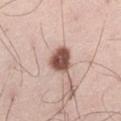Clinical impression:
Imaged during a routine full-body skin examination; the lesion was not biopsied and no histopathology is available.
Context:
Automated tile analysis of the lesion measured a lesion area of about 8 mm² and a shape eccentricity near 0.25. It also reported border irregularity of about 2 on a 0–10 scale, a within-lesion color-variation index near 4/10, and radial color variation of about 1.5. The analysis additionally found a classifier nevus-likeness of about 90/100 and lesion-presence confidence of about 100/100. Cropped from a total-body skin-imaging series; the visible field is about 15 mm. Captured under white-light illumination. The patient is a male aged approximately 35. From the left thigh.